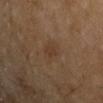workup: no biopsy performed (imaged during a skin exam) | acquisition: total-body-photography crop, ~15 mm field of view | subject: male, about 55 years old | image-analysis metrics: a border-irregularity index near 2.5/10, a color-variation rating of about 2/10, and radial color variation of about 0.5; an automated nevus-likeness rating near 5 out of 100 and lesion-presence confidence of about 100/100 | lesion diameter: ~3.5 mm (longest diameter) | location: the left upper arm | tile lighting: cross-polarized.